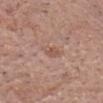Q: Was a biopsy performed?
A: total-body-photography surveillance lesion; no biopsy
Q: How was this image acquired?
A: total-body-photography crop, ~15 mm field of view
Q: Lesion location?
A: the chest
Q: Who is the patient?
A: male, roughly 70 years of age